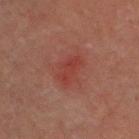  biopsy_status: not biopsied; imaged during a skin examination
  site: head or neck
  image:
    source: total-body photography crop
    field_of_view_mm: 15
  patient:
    sex: male
    age_approx: 70